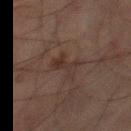  biopsy_status: not biopsied; imaged during a skin examination